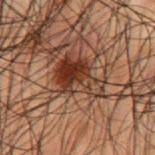notes: catalogued during a skin exam; not biopsied | subject: male, roughly 50 years of age | anatomic site: the back | image-analysis metrics: an area of roughly 15 mm² and a shape-asymmetry score of about 0.4 (0 = symmetric); a nevus-likeness score of about 95/100 and a detector confidence of about 100 out of 100 that the crop contains a lesion | image: ~15 mm crop, total-body skin-cancer survey.